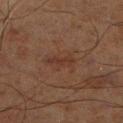This lesion was catalogued during total-body skin photography and was not selected for biopsy.